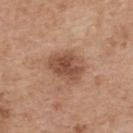Impression:
Imaged during a routine full-body skin examination; the lesion was not biopsied and no histopathology is available.
Acquisition and patient details:
From the upper back. A lesion tile, about 15 mm wide, cut from a 3D total-body photograph. The patient is a female aged 38–42.